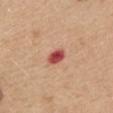Assessment:
No biopsy was performed on this lesion — it was imaged during a full skin examination and was not determined to be concerning.
Context:
The tile uses white-light illumination. A female patient, about 45 years old. A 15 mm crop from a total-body photograph taken for skin-cancer surveillance. Located on the mid back. The recorded lesion diameter is about 2.5 mm. The lesion-visualizer software estimated an average lesion color of about L≈52 a*≈34 b*≈29 (CIELAB), about 16 CIELAB-L* units darker than the surrounding skin, and a normalized lesion–skin contrast near 11. And it measured a color-variation rating of about 4.5/10 and radial color variation of about 1. It also reported a nevus-likeness score of about 0/100.